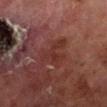{"biopsy_status": "not biopsied; imaged during a skin examination", "site": "left lower leg", "lighting": "cross-polarized", "automated_metrics": {"nevus_likeness_0_100": 0, "lesion_detection_confidence_0_100": 90}, "lesion_size": {"long_diameter_mm_approx": 4.5}, "image": {"source": "total-body photography crop", "field_of_view_mm": 15}, "patient": {"sex": "male", "age_approx": 70}}